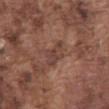Impression:
This lesion was catalogued during total-body skin photography and was not selected for biopsy.
Background:
From the abdomen. A male patient, approximately 75 years of age. This image is a 15 mm lesion crop taken from a total-body photograph.